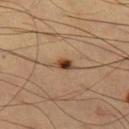Impression: The lesion was photographed on a routine skin check and not biopsied; there is no pathology result. Clinical summary: On the left thigh. A male subject, aged 58–62. A 15 mm close-up extracted from a 3D total-body photography capture.